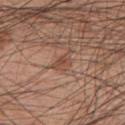Impression: This lesion was catalogued during total-body skin photography and was not selected for biopsy. Background: The tile uses white-light illumination. The total-body-photography lesion software estimated a footprint of about 3.5 mm², an outline eccentricity of about 0.85 (0 = round, 1 = elongated), and a symmetry-axis asymmetry near 0.3. It also reported an average lesion color of about L≈48 a*≈21 b*≈28 (CIELAB), roughly 8 lightness units darker than nearby skin, and a normalized border contrast of about 6. The software also gave a border-irregularity rating of about 3/10 and peripheral color asymmetry of about 0.5. Cropped from a total-body skin-imaging series; the visible field is about 15 mm. The lesion is located on the chest. The subject is a male aged around 60. The recorded lesion diameter is about 3 mm.